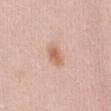Recorded during total-body skin imaging; not selected for excision or biopsy. The lesion is on the abdomen. The subject is a female aged 43 to 47. This is a white-light tile. Longest diameter approximately 3 mm. A region of skin cropped from a whole-body photographic capture, roughly 15 mm wide.